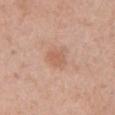| field | value |
|---|---|
| workup | total-body-photography surveillance lesion; no biopsy |
| subject | female, aged around 45 |
| lesion diameter | ≈3.5 mm |
| tile lighting | white-light |
| image source | ~15 mm crop, total-body skin-cancer survey |
| body site | the right upper arm |